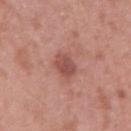Assessment: This lesion was catalogued during total-body skin photography and was not selected for biopsy. Image and clinical context: The recorded lesion diameter is about 2.5 mm. A region of skin cropped from a whole-body photographic capture, roughly 15 mm wide. This is a white-light tile. Automated image analysis of the tile measured about 10 CIELAB-L* units darker than the surrounding skin and a lesion-to-skin contrast of about 7 (normalized; higher = more distinct). The analysis additionally found radial color variation of about 0.5. It also reported a classifier nevus-likeness of about 45/100 and a detector confidence of about 100 out of 100 that the crop contains a lesion. Located on the right upper arm. A female patient, aged 38–42.